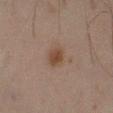Captured during whole-body skin photography for melanoma surveillance; the lesion was not biopsied. Located on the right lower leg. Cropped from a whole-body photographic skin survey; the tile spans about 15 mm. Captured under cross-polarized illumination. A male subject in their mid- to late 40s. The lesion's longest dimension is about 2.5 mm.